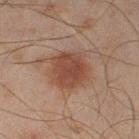biopsy status=no biopsy performed (imaged during a skin exam) | location=the left lower leg | subject=male, aged 43 to 47 | image=15 mm crop, total-body photography | tile lighting=cross-polarized.